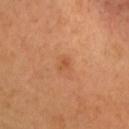Impression:
This lesion was catalogued during total-body skin photography and was not selected for biopsy.
Context:
This image is a 15 mm lesion crop taken from a total-body photograph. This is a cross-polarized tile. Automated image analysis of the tile measured a footprint of about 1.5 mm², an outline eccentricity of about 0.75 (0 = round, 1 = elongated), and two-axis asymmetry of about 0.25. It also reported a lesion color around L≈53 a*≈26 b*≈39 in CIELAB, roughly 7 lightness units darker than nearby skin, and a lesion-to-skin contrast of about 5.5 (normalized; higher = more distinct). The software also gave a border-irregularity index near 2/10, a within-lesion color-variation index near 0/10, and peripheral color asymmetry of about 0. And it measured lesion-presence confidence of about 100/100. From the head or neck. The patient is a female about 40 years old.